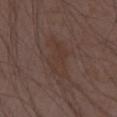Notes:
– notes: total-body-photography surveillance lesion; no biopsy
– image-analysis metrics: a border-irregularity index near 5/10, a within-lesion color-variation index near 2.5/10, and radial color variation of about 1; a nevus-likeness score of about 0/100
– anatomic site: the left upper arm
– tile lighting: white-light
– image: 15 mm crop, total-body photography
– patient: male, about 50 years old
– size: about 5 mm